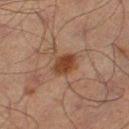| key | value |
|---|---|
| notes | imaged on a skin check; not biopsied |
| subject | male, aged around 70 |
| anatomic site | the left thigh |
| imaging modality | ~15 mm tile from a whole-body skin photo |